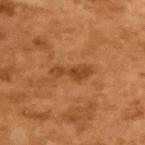A male patient in their mid- to late 60s. A 15 mm close-up tile from a total-body photography series done for melanoma screening. Approximately 4.5 mm at its widest.